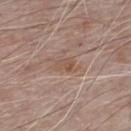No biopsy was performed on this lesion — it was imaged during a full skin examination and was not determined to be concerning. This is a white-light tile. The lesion is on the chest. A 15 mm close-up tile from a total-body photography series done for melanoma screening. The subject is a male roughly 70 years of age.